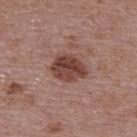Captured during whole-body skin photography for melanoma surveillance; the lesion was not biopsied.
Cropped from a whole-body photographic skin survey; the tile spans about 15 mm.
Imaged with white-light lighting.
The lesion is located on the upper back.
The lesion-visualizer software estimated an average lesion color of about L≈42 a*≈22 b*≈24 (CIELAB), roughly 13 lightness units darker than nearby skin, and a normalized border contrast of about 10. And it measured a border-irregularity index near 2/10 and a color-variation rating of about 5/10. The analysis additionally found a nevus-likeness score of about 55/100 and a lesion-detection confidence of about 100/100.
A female subject approximately 65 years of age.
Measured at roughly 4 mm in maximum diameter.